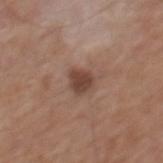Case summary:
– biopsy status — no biopsy performed (imaged during a skin exam)
– lesion diameter — about 2.5 mm
– acquisition — total-body-photography crop, ~15 mm field of view
– anatomic site — the back
– tile lighting — white-light
– subject — male, about 65 years old